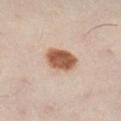Imaged during a routine full-body skin examination; the lesion was not biopsied and no histopathology is available. Located on the left leg. The lesion's longest dimension is about 3.5 mm. An algorithmic analysis of the crop reported an area of roughly 9.5 mm², a shape eccentricity near 0.5, and two-axis asymmetry of about 0.15. The software also gave an automated nevus-likeness rating near 100 out of 100 and lesion-presence confidence of about 100/100. A region of skin cropped from a whole-body photographic capture, roughly 15 mm wide. Captured under cross-polarized illumination. A female patient aged 28 to 32.